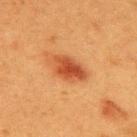Q: Is there a histopathology result?
A: no biopsy performed (imaged during a skin exam)
Q: Who is the patient?
A: female, aged approximately 40
Q: What kind of image is this?
A: ~15 mm tile from a whole-body skin photo
Q: Lesion location?
A: the upper back